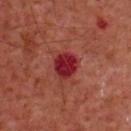This lesion was catalogued during total-body skin photography and was not selected for biopsy. A male patient, aged 58 to 62. The lesion is on the chest. A close-up tile cropped from a whole-body skin photograph, about 15 mm across.